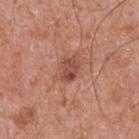{
  "biopsy_status": "not biopsied; imaged during a skin examination",
  "lighting": "white-light",
  "lesion_size": {
    "long_diameter_mm_approx": 3.0
  },
  "patient": {
    "sex": "male",
    "age_approx": 55
  },
  "site": "back",
  "automated_metrics": {
    "cielab_L": 49,
    "cielab_a": 25,
    "cielab_b": 29,
    "vs_skin_darker_L": 10.0,
    "vs_skin_contrast_norm": 7.0,
    "border_irregularity_0_10": 2.5,
    "color_variation_0_10": 4.0,
    "peripheral_color_asymmetry": 1.5,
    "nevus_likeness_0_100": 50,
    "lesion_detection_confidence_0_100": 100
  },
  "image": {
    "source": "total-body photography crop",
    "field_of_view_mm": 15
  }
}